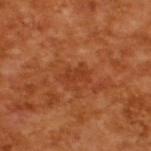workup=imaged on a skin check; not biopsied
patient=male, aged around 65
lesion diameter=~3 mm (longest diameter)
illumination=cross-polarized
imaging modality=~15 mm tile from a whole-body skin photo